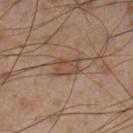Impression: No biopsy was performed on this lesion — it was imaged during a full skin examination and was not determined to be concerning. Image and clinical context: Automated tile analysis of the lesion measured a border-irregularity rating of about 2.5/10 and internal color variation of about 3 on a 0–10 scale. And it measured an automated nevus-likeness rating near 5 out of 100 and a detector confidence of about 100 out of 100 that the crop contains a lesion. On the leg. A male subject aged around 55. The tile uses cross-polarized illumination. Measured at roughly 3.5 mm in maximum diameter. Cropped from a whole-body photographic skin survey; the tile spans about 15 mm.